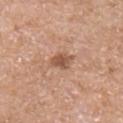Q: Is there a histopathology result?
A: no biopsy performed (imaged during a skin exam)
Q: Where on the body is the lesion?
A: the right upper arm
Q: Illumination type?
A: white-light
Q: What kind of image is this?
A: total-body-photography crop, ~15 mm field of view
Q: Who is the patient?
A: male, roughly 55 years of age